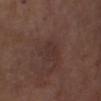biopsy_status: not biopsied; imaged during a skin examination